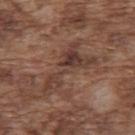| field | value |
|---|---|
| workup | total-body-photography surveillance lesion; no biopsy |
| automated lesion analysis | a lesion area of about 12 mm², an eccentricity of roughly 0.9, and a symmetry-axis asymmetry near 0.7; a normalized border contrast of about 8; a border-irregularity index near 10/10, a color-variation rating of about 5/10, and peripheral color asymmetry of about 1.5; a detector confidence of about 60 out of 100 that the crop contains a lesion |
| acquisition | total-body-photography crop, ~15 mm field of view |
| patient | male, approximately 75 years of age |
| anatomic site | the back |
| lesion size | ≈7.5 mm |